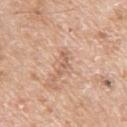Q: Lesion size?
A: ~3 mm (longest diameter)
Q: What are the patient's age and sex?
A: male, in their mid- to late 60s
Q: Lesion location?
A: the arm
Q: What did automated image analysis measure?
A: an average lesion color of about L≈61 a*≈21 b*≈31 (CIELAB), a lesion–skin lightness drop of about 9, and a lesion-to-skin contrast of about 5.5 (normalized; higher = more distinct); lesion-presence confidence of about 100/100
Q: What lighting was used for the tile?
A: white-light
Q: What kind of image is this?
A: ~15 mm crop, total-body skin-cancer survey